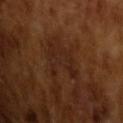No biopsy was performed on this lesion — it was imaged during a full skin examination and was not determined to be concerning. An algorithmic analysis of the crop reported an average lesion color of about L≈23 a*≈18 b*≈25 (CIELAB) and a lesion-to-skin contrast of about 5.5 (normalized; higher = more distinct). And it measured a border-irregularity index near 7/10, a within-lesion color-variation index near 1.5/10, and radial color variation of about 0.5. The analysis additionally found a nevus-likeness score of about 0/100. The lesion's longest dimension is about 4.5 mm. A male subject, aged approximately 65. A lesion tile, about 15 mm wide, cut from a 3D total-body photograph.